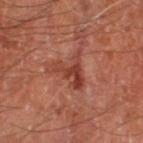- follow-up — total-body-photography surveillance lesion; no biopsy
- subject — male, roughly 70 years of age
- acquisition — 15 mm crop, total-body photography
- anatomic site — the right thigh